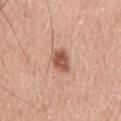Captured during whole-body skin photography for melanoma surveillance; the lesion was not biopsied.
Imaged with white-light lighting.
Cropped from a total-body skin-imaging series; the visible field is about 15 mm.
The lesion's longest dimension is about 3 mm.
A male patient, aged 53 to 57.
The lesion is located on the front of the torso.